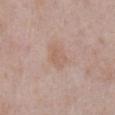follow-up: imaged on a skin check; not biopsied
illumination: white-light
anatomic site: the abdomen
TBP lesion metrics: an average lesion color of about L≈60 a*≈17 b*≈27 (CIELAB) and a lesion-to-skin contrast of about 5 (normalized; higher = more distinct); a border-irregularity index near 2.5/10 and peripheral color asymmetry of about 0.5
patient: male, aged 53 to 57
image: ~15 mm tile from a whole-body skin photo